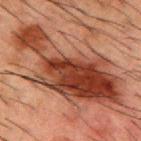Recorded during total-body skin imaging; not selected for excision or biopsy. A 15 mm close-up tile from a total-body photography series done for melanoma screening. About 14 mm across. This is a cross-polarized tile. Automated tile analysis of the lesion measured a border-irregularity index near 7/10, a color-variation rating of about 8.5/10, and peripheral color asymmetry of about 3. From the back. The subject is a male about 50 years old.